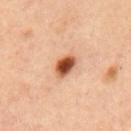Assessment:
This lesion was catalogued during total-body skin photography and was not selected for biopsy.
Context:
A female subject in their mid-40s. The lesion's longest dimension is about 3 mm. The tile uses cross-polarized illumination. From the upper back. This image is a 15 mm lesion crop taken from a total-body photograph.